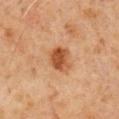workup: no biopsy performed (imaged during a skin exam).